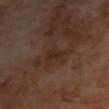This lesion was catalogued during total-body skin photography and was not selected for biopsy.
The lesion is located on the chest.
A 15 mm close-up tile from a total-body photography series done for melanoma screening.
A male patient aged approximately 70.